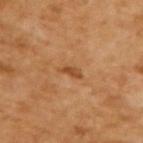Q: Was a biopsy performed?
A: total-body-photography surveillance lesion; no biopsy
Q: What is the imaging modality?
A: ~15 mm tile from a whole-body skin photo
Q: What are the patient's age and sex?
A: male, aged approximately 65
Q: Lesion size?
A: ~2.5 mm (longest diameter)